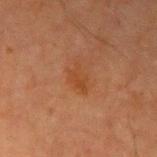The lesion was photographed on a routine skin check and not biopsied; there is no pathology result.
This is a cross-polarized tile.
A 15 mm close-up extracted from a 3D total-body photography capture.
Located on the right upper arm.
The patient is a male aged 63–67.